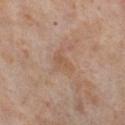workup: total-body-photography surveillance lesion; no biopsy | subject: female, in their mid- to late 50s | image source: ~15 mm crop, total-body skin-cancer survey | TBP lesion metrics: a footprint of about 4 mm², a shape eccentricity near 0.8, and a symmetry-axis asymmetry near 0.4; a border-irregularity rating of about 4/10 and internal color variation of about 1.5 on a 0–10 scale; a nevus-likeness score of about 0/100 and a detector confidence of about 100 out of 100 that the crop contains a lesion | location: the left thigh | diameter: ≈3 mm.